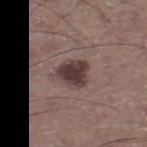notes: catalogued during a skin exam; not biopsied
patient: male, aged around 65
automated metrics: an area of roughly 8 mm², an eccentricity of roughly 0.45, and two-axis asymmetry of about 0.3; a lesion color around L≈37 a*≈15 b*≈17 in CIELAB and a lesion-to-skin contrast of about 11.5 (normalized; higher = more distinct); border irregularity of about 2.5 on a 0–10 scale, a color-variation rating of about 3/10, and peripheral color asymmetry of about 0.5
acquisition: ~15 mm crop, total-body skin-cancer survey
illumination: white-light
lesion size: ~3.5 mm (longest diameter)
body site: the leg